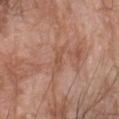biopsy status: total-body-photography surveillance lesion; no biopsy | illumination: white-light illumination | TBP lesion metrics: a nevus-likeness score of about 0/100 | patient: male, aged 58 to 62 | diameter: about 2.5 mm | acquisition: ~15 mm tile from a whole-body skin photo | location: the left forearm.